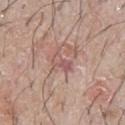| field | value |
|---|---|
| notes | total-body-photography surveillance lesion; no biopsy |
| imaging modality | 15 mm crop, total-body photography |
| patient | male, about 65 years old |
| location | the chest |